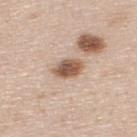Clinical impression: Recorded during total-body skin imaging; not selected for excision or biopsy. Background: Automated image analysis of the tile measured an average lesion color of about L≈56 a*≈18 b*≈29 (CIELAB), a lesion–skin lightness drop of about 16, and a normalized lesion–skin contrast near 10. The software also gave a border-irregularity index near 1.5/10, a within-lesion color-variation index near 6/10, and a peripheral color-asymmetry measure near 2. A 15 mm close-up tile from a total-body photography series done for melanoma screening. About 3.5 mm across. The lesion is on the upper back. A male subject aged around 45.